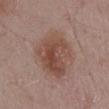Assessment: Captured during whole-body skin photography for melanoma surveillance; the lesion was not biopsied. Acquisition and patient details: From the mid back. A lesion tile, about 15 mm wide, cut from a 3D total-body photograph. The subject is a male aged 53 to 57. The tile uses white-light illumination. The recorded lesion diameter is about 7 mm.